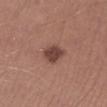Assessment:
Imaged during a routine full-body skin examination; the lesion was not biopsied and no histopathology is available.
Image and clinical context:
From the right lower leg. The recorded lesion diameter is about 3 mm. The patient is a male aged around 40. The tile uses white-light illumination. A lesion tile, about 15 mm wide, cut from a 3D total-body photograph. Automated image analysis of the tile measured an eccentricity of roughly 0.7. It also reported a border-irregularity rating of about 2/10, a within-lesion color-variation index near 1.5/10, and a peripheral color-asymmetry measure near 0.5.